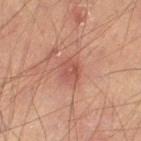| feature | finding |
|---|---|
| workup | catalogued during a skin exam; not biopsied |
| image | ~15 mm crop, total-body skin-cancer survey |
| location | the right lower leg |
| tile lighting | cross-polarized illumination |
| subject | male, roughly 50 years of age |